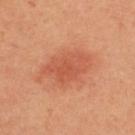{"biopsy_status": "not biopsied; imaged during a skin examination", "automated_metrics": {"area_mm2_approx": 13.0, "eccentricity": 0.8, "shape_asymmetry": 0.25, "border_irregularity_0_10": 3.0, "peripheral_color_asymmetry": 1.0}, "image": {"source": "total-body photography crop", "field_of_view_mm": 15}, "site": "upper back", "lighting": "cross-polarized", "patient": {"sex": "female", "age_approx": 45}, "lesion_size": {"long_diameter_mm_approx": 5.5}}